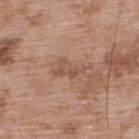<tbp_lesion>
  <biopsy_status>not biopsied; imaged during a skin examination</biopsy_status>
  <site>upper back</site>
  <automated_metrics>
    <area_mm2_approx>5.5</area_mm2_approx>
    <eccentricity>0.9</eccentricity>
    <cielab_L>52</cielab_L>
    <cielab_a>20</cielab_a>
    <cielab_b>30</cielab_b>
    <vs_skin_darker_L>8.0</vs_skin_darker_L>
    <border_irregularity_0_10>6.5</border_irregularity_0_10>
    <color_variation_0_10>2.5</color_variation_0_10>
    <peripheral_color_asymmetry>0.5</peripheral_color_asymmetry>
    <nevus_likeness_0_100>0</nevus_likeness_0_100>
    <lesion_detection_confidence_0_100>100</lesion_detection_confidence_0_100>
  </automated_metrics>
  <lighting>white-light</lighting>
  <patient>
    <sex>male</sex>
    <age_approx>50</age_approx>
  </patient>
  <image>
    <source>total-body photography crop</source>
    <field_of_view_mm>15</field_of_view_mm>
  </image>
  <lesion_size>
    <long_diameter_mm_approx>4.0</long_diameter_mm_approx>
  </lesion_size>
</tbp_lesion>